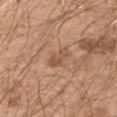- workup: imaged on a skin check; not biopsied
- TBP lesion metrics: an area of roughly 4 mm², a shape eccentricity near 0.85, and a shape-asymmetry score of about 0.35 (0 = symmetric); border irregularity of about 4.5 on a 0–10 scale, internal color variation of about 1.5 on a 0–10 scale, and a peripheral color-asymmetry measure near 0.5
- acquisition: 15 mm crop, total-body photography
- illumination: white-light illumination
- location: the left upper arm
- patient: male, aged 48–52